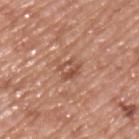Captured during whole-body skin photography for melanoma surveillance; the lesion was not biopsied.
The recorded lesion diameter is about 2.5 mm.
Cropped from a whole-body photographic skin survey; the tile spans about 15 mm.
Imaged with white-light lighting.
A male subject, approximately 50 years of age.
The lesion is located on the upper back.